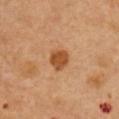notes: total-body-photography surveillance lesion; no biopsy.